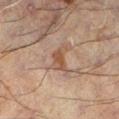No biopsy was performed on this lesion — it was imaged during a full skin examination and was not determined to be concerning. A male patient, aged approximately 60. From the left lower leg. A roughly 15 mm field-of-view crop from a total-body skin photograph. This is a cross-polarized tile. The lesion's longest dimension is about 3 mm.